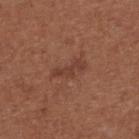No biopsy was performed on this lesion — it was imaged during a full skin examination and was not determined to be concerning. The recorded lesion diameter is about 4 mm. Located on the upper back. A female patient, approximately 65 years of age. Cropped from a whole-body photographic skin survey; the tile spans about 15 mm.